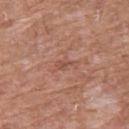{
  "biopsy_status": "not biopsied; imaged during a skin examination",
  "site": "chest",
  "patient": {
    "sex": "male",
    "age_approx": 60
  },
  "image": {
    "source": "total-body photography crop",
    "field_of_view_mm": 15
  },
  "lighting": "white-light",
  "automated_metrics": {
    "area_mm2_approx": 2.5,
    "eccentricity": 0.9,
    "border_irregularity_0_10": 4.5,
    "peripheral_color_asymmetry": 0.0,
    "nevus_likeness_0_100": 0,
    "lesion_detection_confidence_0_100": 100
  },
  "lesion_size": {
    "long_diameter_mm_approx": 2.5
  }
}